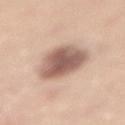biopsy status: catalogued during a skin exam; not biopsied.